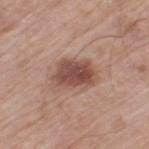notes: catalogued during a skin exam; not biopsied
acquisition: total-body-photography crop, ~15 mm field of view
diameter: ≈4.5 mm
subject: male, about 80 years old
tile lighting: white-light
anatomic site: the left thigh
automated lesion analysis: a lesion color around L≈49 a*≈21 b*≈25 in CIELAB and a normalized lesion–skin contrast near 9; a border-irregularity index near 2.5/10, a color-variation rating of about 4/10, and peripheral color asymmetry of about 1; a lesion-detection confidence of about 100/100